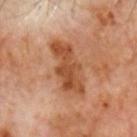Imaged during a routine full-body skin examination; the lesion was not biopsied and no histopathology is available.
The patient is a male approximately 60 years of age.
About 6 mm across.
The lesion-visualizer software estimated an eccentricity of roughly 0.9 and two-axis asymmetry of about 0.25. The software also gave a color-variation rating of about 4.5/10. The analysis additionally found an automated nevus-likeness rating near 0 out of 100.
A 15 mm close-up extracted from a 3D total-body photography capture.
This is a cross-polarized tile.
The lesion is located on the head or neck.